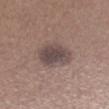The lesion was tiled from a total-body skin photograph and was not biopsied. The tile uses white-light illumination. The lesion-visualizer software estimated a border-irregularity index near 2/10, internal color variation of about 4 on a 0–10 scale, and a peripheral color-asymmetry measure near 1. A roughly 15 mm field-of-view crop from a total-body skin photograph. A male patient about 65 years old. From the leg. Measured at roughly 4.5 mm in maximum diameter.